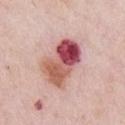The lesion was tiled from a total-body skin photograph and was not biopsied. A female patient in their mid-60s. The total-body-photography lesion software estimated a lesion area of about 19 mm² and a symmetry-axis asymmetry near 0.25. It also reported an average lesion color of about L≈55 a*≈29 b*≈24 (CIELAB), roughly 18 lightness units darker than nearby skin, and a normalized lesion–skin contrast near 11.5. Longest diameter approximately 6 mm. Located on the chest. A 15 mm close-up tile from a total-body photography series done for melanoma screening.